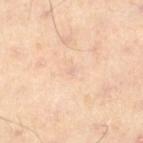  biopsy_status: not biopsied; imaged during a skin examination
  automated_metrics:
    cielab_L: 76
    cielab_a: 19
    cielab_b: 30
    vs_skin_contrast_norm: 3.0
    border_irregularity_0_10: 3.5
    peripheral_color_asymmetry: 0.0
  lesion_size:
    long_diameter_mm_approx: 1.0
  patient:
    sex: male
    age_approx: 55
  site: left lower leg
  lighting: cross-polarized
  image:
    source: total-body photography crop
    field_of_view_mm: 15